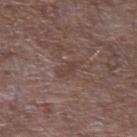Case summary:
* workup · imaged on a skin check; not biopsied